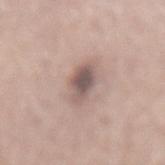• follow-up — total-body-photography surveillance lesion; no biopsy
• tile lighting — white-light illumination
• imaging modality — 15 mm crop, total-body photography
• body site — the lower back
• subject — male, aged around 60